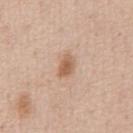Part of a total-body skin-imaging series; this lesion was reviewed on a skin check and was not flagged for biopsy. About 3 mm across. Automated image analysis of the tile measured a shape eccentricity near 0.75 and two-axis asymmetry of about 0.2. It also reported a lesion–skin lightness drop of about 11 and a normalized border contrast of about 7.5. The software also gave a border-irregularity index near 2/10 and a color-variation rating of about 3/10. The software also gave an automated nevus-likeness rating near 75 out of 100. Captured under white-light illumination. This image is a 15 mm lesion crop taken from a total-body photograph. From the abdomen. The patient is a male aged 48–52.